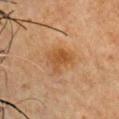Q: Was a biopsy performed?
A: catalogued during a skin exam; not biopsied
Q: Illumination type?
A: cross-polarized
Q: Lesion size?
A: ≈3.5 mm
Q: What is the imaging modality?
A: total-body-photography crop, ~15 mm field of view
Q: Lesion location?
A: the chest
Q: What did automated image analysis measure?
A: a footprint of about 8.5 mm², an outline eccentricity of about 0.6 (0 = round, 1 = elongated), and a symmetry-axis asymmetry near 0.4; border irregularity of about 4 on a 0–10 scale, internal color variation of about 3 on a 0–10 scale, and peripheral color asymmetry of about 1
Q: What are the patient's age and sex?
A: male, in their mid- to late 60s